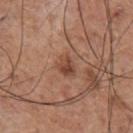Q: Was a biopsy performed?
A: no biopsy performed (imaged during a skin exam)